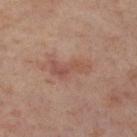<tbp_lesion>
<biopsy_status>not biopsied; imaged during a skin examination</biopsy_status>
<lesion_size>
  <long_diameter_mm_approx>4.5</long_diameter_mm_approx>
</lesion_size>
<image>
  <source>total-body photography crop</source>
  <field_of_view_mm>15</field_of_view_mm>
</image>
<lighting>cross-polarized</lighting>
<site>right lower leg</site>
<patient>
  <sex>female</sex>
  <age_approx>55</age_approx>
</patient>
</tbp_lesion>